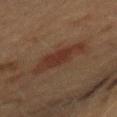{
  "biopsy_status": "not biopsied; imaged during a skin examination",
  "image": {
    "source": "total-body photography crop",
    "field_of_view_mm": 15
  },
  "patient": {
    "sex": "female",
    "age_approx": 60
  },
  "site": "mid back",
  "lesion_size": {
    "long_diameter_mm_approx": 6.5
  }
}